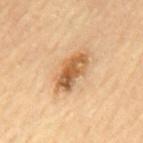Clinical summary:
About 5.5 mm across. Imaged with cross-polarized lighting. A 15 mm close-up extracted from a 3D total-body photography capture. A male subject, aged approximately 85 or older. Located on the back.